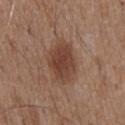Clinical impression:
Captured during whole-body skin photography for melanoma surveillance; the lesion was not biopsied.
Image and clinical context:
Cropped from a whole-body photographic skin survey; the tile spans about 15 mm. The patient is a male in their mid-70s. On the abdomen. This is a white-light tile.